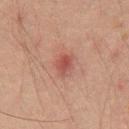Clinical impression: No biopsy was performed on this lesion — it was imaged during a full skin examination and was not determined to be concerning. Context: Longest diameter approximately 2.5 mm. An algorithmic analysis of the crop reported a footprint of about 4 mm² and two-axis asymmetry of about 0.25. It also reported about 8 CIELAB-L* units darker than the surrounding skin and a normalized lesion–skin contrast near 7. It also reported a nevus-likeness score of about 20/100 and a detector confidence of about 100 out of 100 that the crop contains a lesion. Located on the chest. The patient is a male in their mid-40s. A lesion tile, about 15 mm wide, cut from a 3D total-body photograph.